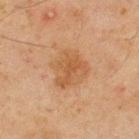Impression: This lesion was catalogued during total-body skin photography and was not selected for biopsy. Clinical summary: The lesion is on the upper back. A male patient, aged around 45. A 15 mm close-up tile from a total-body photography series done for melanoma screening.